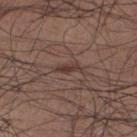Clinical impression:
This lesion was catalogued during total-body skin photography and was not selected for biopsy.
Image and clinical context:
A region of skin cropped from a whole-body photographic capture, roughly 15 mm wide. Approximately 2.5 mm at its widest. Automated image analysis of the tile measured a lesion area of about 2 mm², a shape eccentricity near 0.9, and a symmetry-axis asymmetry near 0.35. The analysis additionally found about 8 CIELAB-L* units darker than the surrounding skin and a normalized border contrast of about 7.5. The analysis additionally found a nevus-likeness score of about 20/100 and lesion-presence confidence of about 80/100. The patient is a male approximately 65 years of age. From the left lower leg. Captured under white-light illumination.